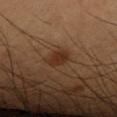Part of a total-body skin-imaging series; this lesion was reviewed on a skin check and was not flagged for biopsy.
From the right upper arm.
A 15 mm close-up tile from a total-body photography series done for melanoma screening.
The patient is a male roughly 60 years of age.
The lesion's longest dimension is about 3 mm.